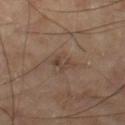Captured during whole-body skin photography for melanoma surveillance; the lesion was not biopsied.
Measured at roughly 3.5 mm in maximum diameter.
This is a cross-polarized tile.
A 15 mm crop from a total-body photograph taken for skin-cancer surveillance.
A male subject, aged approximately 65.
Located on the left thigh.